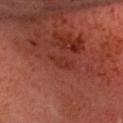Notes:
– notes · no biopsy performed (imaged during a skin exam)
– image source · ~15 mm tile from a whole-body skin photo
– site · the head or neck
– patient · male, aged 58 to 62
– illumination · cross-polarized
– lesion diameter · about 2.5 mm